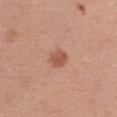biopsy_status: not biopsied; imaged during a skin examination